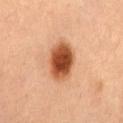{"biopsy_status": "not biopsied; imaged during a skin examination"}